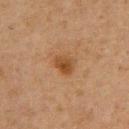Clinical impression: The lesion was tiled from a total-body skin photograph and was not biopsied. Context: Located on the chest. This image is a 15 mm lesion crop taken from a total-body photograph. A male patient, aged 73–77. This is a cross-polarized tile. The lesion-visualizer software estimated a mean CIELAB color near L≈38 a*≈18 b*≈31 and roughly 8 lightness units darker than nearby skin. It also reported a border-irregularity rating of about 2.5/10, internal color variation of about 3.5 on a 0–10 scale, and peripheral color asymmetry of about 1.